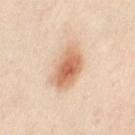biopsy status = no biopsy performed (imaged during a skin exam)
image-analysis metrics = a footprint of about 14 mm², an eccentricity of roughly 0.85, and two-axis asymmetry of about 0.15; a lesion color around L≈68 a*≈21 b*≈34 in CIELAB, about 14 CIELAB-L* units darker than the surrounding skin, and a normalized border contrast of about 8.5; a border-irregularity rating of about 2/10, internal color variation of about 6.5 on a 0–10 scale, and radial color variation of about 1.5; a nevus-likeness score of about 95/100 and a lesion-detection confidence of about 100/100
subject = female, aged 38–42
tile lighting = cross-polarized illumination
body site = the right leg
image source = ~15 mm tile from a whole-body skin photo